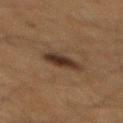The lesion was tiled from a total-body skin photograph and was not biopsied. The lesion is on the mid back. Longest diameter approximately 4 mm. This image is a 15 mm lesion crop taken from a total-body photograph. A male patient roughly 65 years of age. The lesion-visualizer software estimated a lesion area of about 6 mm², an eccentricity of roughly 0.9, and two-axis asymmetry of about 0.2. The analysis additionally found a mean CIELAB color near L≈26 a*≈14 b*≈22, roughly 11 lightness units darker than nearby skin, and a normalized lesion–skin contrast near 11.5. Imaged with cross-polarized lighting.